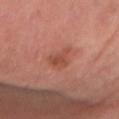workup: catalogued during a skin exam; not biopsied
anatomic site: the right forearm
lighting: cross-polarized
image: total-body-photography crop, ~15 mm field of view
lesion diameter: about 3.5 mm
patient: female, aged 63 to 67